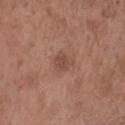Notes:
– biopsy status: catalogued during a skin exam; not biopsied
– image: 15 mm crop, total-body photography
– site: the head or neck
– patient: male, aged 33–37